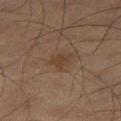Part of a total-body skin-imaging series; this lesion was reviewed on a skin check and was not flagged for biopsy.
The recorded lesion diameter is about 2.5 mm.
This image is a 15 mm lesion crop taken from a total-body photograph.
The patient is a male approximately 65 years of age.
On the right thigh.